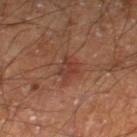No biopsy was performed on this lesion — it was imaged during a full skin examination and was not determined to be concerning. Measured at roughly 3 mm in maximum diameter. A male subject approximately 60 years of age. A roughly 15 mm field-of-view crop from a total-body skin photograph. Imaged with cross-polarized lighting. Automated image analysis of the tile measured a footprint of about 4.5 mm² and two-axis asymmetry of about 0.3. It also reported a mean CIELAB color near L≈35 a*≈22 b*≈25, about 6 CIELAB-L* units darker than the surrounding skin, and a lesion-to-skin contrast of about 6 (normalized; higher = more distinct). On the right thigh.